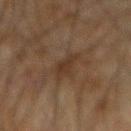No biopsy was performed on this lesion — it was imaged during a full skin examination and was not determined to be concerning. Captured under cross-polarized illumination. The lesion is located on the arm. The recorded lesion diameter is about 3 mm. The subject is a male about 45 years old. This image is a 15 mm lesion crop taken from a total-body photograph. Automated tile analysis of the lesion measured peripheral color asymmetry of about 1.